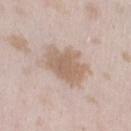Clinical impression: This lesion was catalogued during total-body skin photography and was not selected for biopsy. Context: A region of skin cropped from a whole-body photographic capture, roughly 15 mm wide. A female patient, aged approximately 25. Located on the left thigh.